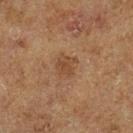No biopsy was performed on this lesion — it was imaged during a full skin examination and was not determined to be concerning. Captured under cross-polarized illumination. A 15 mm close-up tile from a total-body photography series done for melanoma screening. A male patient, roughly 75 years of age. From the left lower leg. Measured at roughly 2.5 mm in maximum diameter.